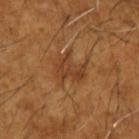Imaged during a routine full-body skin examination; the lesion was not biopsied and no histopathology is available. Imaged with cross-polarized lighting. The subject is a male approximately 65 years of age. Longest diameter approximately 4.5 mm. From the arm. The total-body-photography lesion software estimated a lesion color around L≈41 a*≈22 b*≈35 in CIELAB. It also reported a nevus-likeness score of about 10/100 and a lesion-detection confidence of about 100/100. A lesion tile, about 15 mm wide, cut from a 3D total-body photograph.